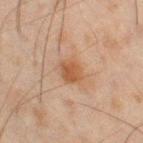Clinical impression: The lesion was photographed on a routine skin check and not biopsied; there is no pathology result. Image and clinical context: The lesion-visualizer software estimated a classifier nevus-likeness of about 85/100 and a detector confidence of about 100 out of 100 that the crop contains a lesion. On the right thigh. About 3 mm across. Cropped from a whole-body photographic skin survey; the tile spans about 15 mm. A male subject, about 45 years old.